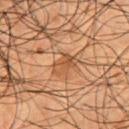Q: Is there a histopathology result?
A: catalogued during a skin exam; not biopsied
Q: What is the imaging modality?
A: total-body-photography crop, ~15 mm field of view
Q: What is the anatomic site?
A: the chest
Q: How was the tile lit?
A: cross-polarized
Q: Lesion size?
A: ≈3.5 mm
Q: What are the patient's age and sex?
A: male, in their mid- to late 50s